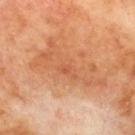Assessment: Captured during whole-body skin photography for melanoma surveillance; the lesion was not biopsied. Context: Automated tile analysis of the lesion measured a color-variation rating of about 4.5/10 and a peripheral color-asymmetry measure near 1.5. It also reported a nevus-likeness score of about 0/100. A male subject aged approximately 70. A lesion tile, about 15 mm wide, cut from a 3D total-body photograph. Located on the upper back. Imaged with cross-polarized lighting.